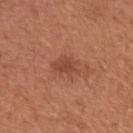{"biopsy_status": "not biopsied; imaged during a skin examination", "image": {"source": "total-body photography crop", "field_of_view_mm": 15}, "patient": {"sex": "female", "age_approx": 65}, "lesion_size": {"long_diameter_mm_approx": 3.5}, "site": "left upper arm", "lighting": "white-light"}